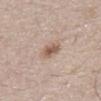Q: Was this lesion biopsied?
A: total-body-photography surveillance lesion; no biopsy